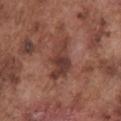Q: Was a biopsy performed?
A: total-body-photography surveillance lesion; no biopsy
Q: What is the imaging modality?
A: ~15 mm tile from a whole-body skin photo
Q: Where on the body is the lesion?
A: the chest
Q: Who is the patient?
A: male, in their mid- to late 70s